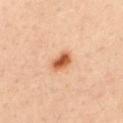follow-up: imaged on a skin check; not biopsied
lesion diameter: about 3 mm
subject: male, aged 33–37
body site: the upper back
lighting: cross-polarized illumination
imaging modality: 15 mm crop, total-body photography
image-analysis metrics: an average lesion color of about L≈58 a*≈27 b*≈38 (CIELAB) and a lesion–skin lightness drop of about 15; an automated nevus-likeness rating near 100 out of 100